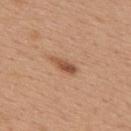biopsy status: total-body-photography surveillance lesion; no biopsy
lesion size: about 3 mm
tile lighting: white-light illumination
imaging modality: 15 mm crop, total-body photography
TBP lesion metrics: a lesion area of about 3.5 mm², an outline eccentricity of about 0.9 (0 = round, 1 = elongated), and a symmetry-axis asymmetry near 0.35; a border-irregularity index near 3.5/10, internal color variation of about 2 on a 0–10 scale, and a peripheral color-asymmetry measure near 0.5; an automated nevus-likeness rating near 75 out of 100
patient: female, aged around 40
location: the upper back